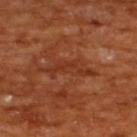<case>
  <biopsy_status>not biopsied; imaged during a skin examination</biopsy_status>
  <image>
    <source>total-body photography crop</source>
    <field_of_view_mm>15</field_of_view_mm>
  </image>
  <site>upper back</site>
  <patient>
    <sex>male</sex>
    <age_approx>65</age_approx>
  </patient>
  <lesion_size>
    <long_diameter_mm_approx>6.5</long_diameter_mm_approx>
  </lesion_size>
  <automated_metrics>
    <eccentricity>0.95</eccentricity>
    <shape_asymmetry>0.6</shape_asymmetry>
    <nevus_likeness_0_100>0</nevus_likeness_0_100>
    <lesion_detection_confidence_0_100>85</lesion_detection_confidence_0_100>
  </automated_metrics>
  <lighting>cross-polarized</lighting>
</case>